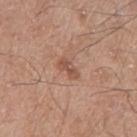patient: male, aged 78 to 82; imaging modality: 15 mm crop, total-body photography; location: the left thigh; illumination: white-light; lesion diameter: about 3 mm.